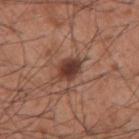Cropped from a whole-body photographic skin survey; the tile spans about 15 mm.
Measured at roughly 3.5 mm in maximum diameter.
The tile uses white-light illumination.
The lesion is located on the arm.
A male patient, roughly 60 years of age.